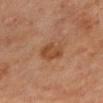Part of a total-body skin-imaging series; this lesion was reviewed on a skin check and was not flagged for biopsy. Imaged with cross-polarized lighting. An algorithmic analysis of the crop reported a footprint of about 7 mm² and a shape-asymmetry score of about 0.2 (0 = symmetric). It also reported a mean CIELAB color near L≈46 a*≈23 b*≈34, about 8 CIELAB-L* units darker than the surrounding skin, and a normalized border contrast of about 7. The software also gave border irregularity of about 2.5 on a 0–10 scale and peripheral color asymmetry of about 0.5. Cropped from a total-body skin-imaging series; the visible field is about 15 mm. The subject is a male aged 68 to 72. The lesion is located on the mid back. Measured at roughly 4 mm in maximum diameter.